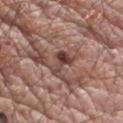Captured during whole-body skin photography for melanoma surveillance; the lesion was not biopsied. Captured under white-light illumination. The lesion is located on the right upper arm. Cropped from a total-body skin-imaging series; the visible field is about 15 mm. Longest diameter approximately 3 mm. The subject is a male aged 63–67.